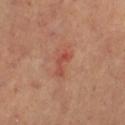workup: imaged on a skin check; not biopsied | patient: female, aged 38–42 | imaging modality: ~15 mm tile from a whole-body skin photo | location: the left thigh.